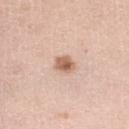workup: imaged on a skin check; not biopsied | automated metrics: a detector confidence of about 100 out of 100 that the crop contains a lesion | image source: total-body-photography crop, ~15 mm field of view | lighting: white-light illumination | anatomic site: the right lower leg | lesion diameter: about 2 mm | patient: female, in their mid-40s.